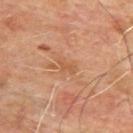Captured during whole-body skin photography for melanoma surveillance; the lesion was not biopsied.
Captured under cross-polarized illumination.
The subject is a male aged 63–67.
This image is a 15 mm lesion crop taken from a total-body photograph.
Located on the upper back.